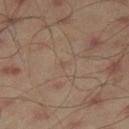Imaged with cross-polarized lighting. Longest diameter approximately 1 mm. Cropped from a whole-body photographic skin survey; the tile spans about 15 mm. From the leg. A male patient in their mid- to late 40s.